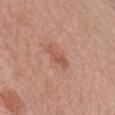follow-up = imaged on a skin check; not biopsied
patient = female, roughly 50 years of age
body site = the chest
image source = ~15 mm crop, total-body skin-cancer survey